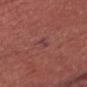lighting: white-light
patient:
  sex: male
  age_approx: 55
site: chest
image:
  source: total-body photography crop
  field_of_view_mm: 15